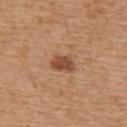Recorded during total-body skin imaging; not selected for excision or biopsy. This is a white-light tile. An algorithmic analysis of the crop reported a footprint of about 5 mm², an outline eccentricity of about 0.75 (0 = round, 1 = elongated), and a shape-asymmetry score of about 0.25 (0 = symmetric). The analysis additionally found an automated nevus-likeness rating near 90 out of 100 and lesion-presence confidence of about 100/100. A lesion tile, about 15 mm wide, cut from a 3D total-body photograph. A male subject roughly 70 years of age. Longest diameter approximately 3 mm. On the upper back.